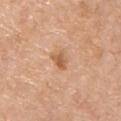Notes:
– notes: total-body-photography surveillance lesion; no biopsy
– patient: male, in their 70s
– TBP lesion metrics: a lesion area of about 3.5 mm², an outline eccentricity of about 0.75 (0 = round, 1 = elongated), and a shape-asymmetry score of about 0.35 (0 = symmetric)
– image: ~15 mm tile from a whole-body skin photo
– anatomic site: the chest
– diameter: ≈2.5 mm
– lighting: white-light